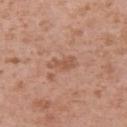Part of a total-body skin-imaging series; this lesion was reviewed on a skin check and was not flagged for biopsy.
The lesion's longest dimension is about 3.5 mm.
On the arm.
The tile uses white-light illumination.
A lesion tile, about 15 mm wide, cut from a 3D total-body photograph.
The subject is a male aged around 40.